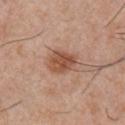Clinical impression: Part of a total-body skin-imaging series; this lesion was reviewed on a skin check and was not flagged for biopsy. Acquisition and patient details: A 15 mm crop from a total-body photograph taken for skin-cancer surveillance. The subject is a male aged approximately 30. The recorded lesion diameter is about 4 mm. On the chest. The lesion-visualizer software estimated a lesion–skin lightness drop of about 11 and a normalized border contrast of about 8.5. And it measured a lesion-detection confidence of about 100/100. This is a white-light tile.